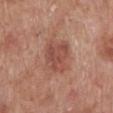Part of a total-body skin-imaging series; this lesion was reviewed on a skin check and was not flagged for biopsy.
A lesion tile, about 15 mm wide, cut from a 3D total-body photograph.
A male patient approximately 70 years of age.
The lesion's longest dimension is about 4 mm.
Imaged with white-light lighting.
Located on the left lower leg.
Automated tile analysis of the lesion measured a lesion area of about 12 mm² and a symmetry-axis asymmetry near 0.2. And it measured a color-variation rating of about 3.5/10. The analysis additionally found an automated nevus-likeness rating near 25 out of 100 and a detector confidence of about 100 out of 100 that the crop contains a lesion.